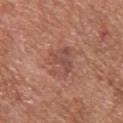image-analysis metrics: a footprint of about 9 mm², an eccentricity of roughly 0.65, and two-axis asymmetry of about 0.25; a border-irregularity index near 4/10 and radial color variation of about 1 | patient: male, aged 73–77 | imaging modality: total-body-photography crop, ~15 mm field of view | diameter: ≈4 mm | site: the back.